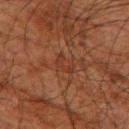follow-up: no biopsy performed (imaged during a skin exam).